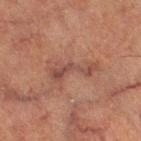biopsy status = catalogued during a skin exam; not biopsied | site = the left thigh | image source = ~15 mm crop, total-body skin-cancer survey | lesion size = about 5.5 mm | patient = male, roughly 65 years of age | tile lighting = cross-polarized | TBP lesion metrics = an eccentricity of roughly 0.95 and two-axis asymmetry of about 0.45; a lesion color around L≈37 a*≈18 b*≈22 in CIELAB and roughly 6 lightness units darker than nearby skin; border irregularity of about 7.5 on a 0–10 scale and peripheral color asymmetry of about 1.